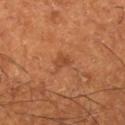<lesion>
  <biopsy_status>not biopsied; imaged during a skin examination</biopsy_status>
  <lighting>cross-polarized</lighting>
  <image>
    <source>total-body photography crop</source>
    <field_of_view_mm>15</field_of_view_mm>
  </image>
  <patient>
    <sex>male</sex>
    <age_approx>65</age_approx>
  </patient>
  <site>right lower leg</site>
  <lesion_size>
    <long_diameter_mm_approx>2.5</long_diameter_mm_approx>
  </lesion_size>
</lesion>